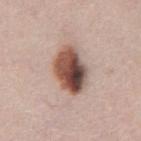workup: imaged on a skin check; not biopsied | image source: total-body-photography crop, ~15 mm field of view | size: ≈5.5 mm | illumination: white-light illumination | site: the chest | subject: male, aged 48–52.